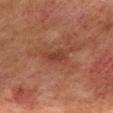Assessment:
The lesion was photographed on a routine skin check and not biopsied; there is no pathology result.
Background:
Captured under cross-polarized illumination. On the mid back. Approximately 3.5 mm at its widest. A region of skin cropped from a whole-body photographic capture, roughly 15 mm wide. A male subject aged 73–77.